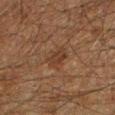Assessment: This lesion was catalogued during total-body skin photography and was not selected for biopsy. Clinical summary: The lesion is on the left lower leg. About 3 mm across. A roughly 15 mm field-of-view crop from a total-body skin photograph. This is a cross-polarized tile. A male patient aged 58–62.